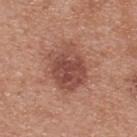Assessment:
The lesion was photographed on a routine skin check and not biopsied; there is no pathology result.
Clinical summary:
On the upper back. The patient is a male roughly 30 years of age. A roughly 15 mm field-of-view crop from a total-body skin photograph. Captured under white-light illumination. The lesion-visualizer software estimated border irregularity of about 2 on a 0–10 scale and a within-lesion color-variation index near 4.5/10. And it measured an automated nevus-likeness rating near 50 out of 100. The lesion's longest dimension is about 5.5 mm.